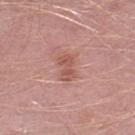The lesion was tiled from a total-body skin photograph and was not biopsied. Cropped from a total-body skin-imaging series; the visible field is about 15 mm. The lesion is on the leg. A male patient approximately 50 years of age. Longest diameter approximately 3 mm.